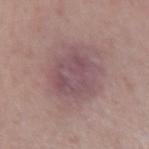The lesion was tiled from a total-body skin photograph and was not biopsied. Cropped from a whole-body photographic skin survey; the tile spans about 15 mm. A female patient, approximately 40 years of age. On the right forearm.